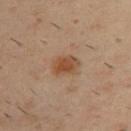imaging modality = ~15 mm tile from a whole-body skin photo
subject = male, aged approximately 40
anatomic site = the upper back